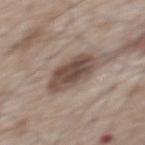follow-up: catalogued during a skin exam; not biopsied | subject: male, roughly 65 years of age | location: the upper back | tile lighting: white-light | automated lesion analysis: a footprint of about 12 mm² and an outline eccentricity of about 0.75 (0 = round, 1 = elongated); a lesion color around L≈47 a*≈15 b*≈22 in CIELAB, roughly 14 lightness units darker than nearby skin, and a lesion-to-skin contrast of about 10 (normalized; higher = more distinct); an automated nevus-likeness rating near 60 out of 100 and a detector confidence of about 100 out of 100 that the crop contains a lesion | size: about 5 mm | acquisition: 15 mm crop, total-body photography.